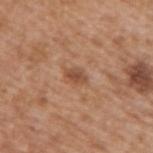* follow-up: no biopsy performed (imaged during a skin exam)
* site: the right upper arm
* patient: male, approximately 65 years of age
* image source: total-body-photography crop, ~15 mm field of view